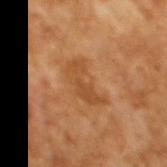Clinical impression: This lesion was catalogued during total-body skin photography and was not selected for biopsy. Acquisition and patient details: The subject is a male approximately 65 years of age. A 15 mm close-up extracted from a 3D total-body photography capture. Captured under cross-polarized illumination. Automated tile analysis of the lesion measured a nevus-likeness score of about 0/100 and a detector confidence of about 100 out of 100 that the crop contains a lesion.